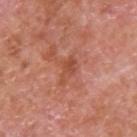<lesion>
<biopsy_status>not biopsied; imaged during a skin examination</biopsy_status>
<patient>
  <sex>male</sex>
  <age_approx>65</age_approx>
</patient>
<image>
  <source>total-body photography crop</source>
  <field_of_view_mm>15</field_of_view_mm>
</image>
<lesion_size>
  <long_diameter_mm_approx>3.0</long_diameter_mm_approx>
</lesion_size>
<site>upper back</site>
<lighting>white-light</lighting>
</lesion>